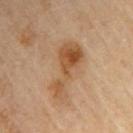  biopsy_status: not biopsied; imaged during a skin examination
  patient:
    sex: male
    age_approx: 85+
  automated_metrics:
    area_mm2_approx: 14.0
    eccentricity: 0.95
    shape_asymmetry: 0.55
    nevus_likeness_0_100: 20
  lesion_size:
    long_diameter_mm_approx: 7.0
  image:
    source: total-body photography crop
    field_of_view_mm: 15
  site: right upper arm